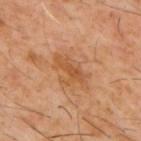{"biopsy_status": "not biopsied; imaged during a skin examination", "automated_metrics": {"border_irregularity_0_10": 6.0, "color_variation_0_10": 2.5, "peripheral_color_asymmetry": 0.5, "nevus_likeness_0_100": 0, "lesion_detection_confidence_0_100": 100}, "site": "upper back", "image": {"source": "total-body photography crop", "field_of_view_mm": 15}, "lighting": "cross-polarized", "patient": {"sex": "male", "age_approx": 60}, "lesion_size": {"long_diameter_mm_approx": 4.5}}